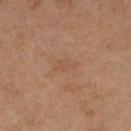follow-up=imaged on a skin check; not biopsied | patient=female, approximately 65 years of age | acquisition=~15 mm tile from a whole-body skin photo | anatomic site=the right lower leg.